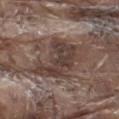The lesion was tiled from a total-body skin photograph and was not biopsied.
On the left thigh.
The tile uses white-light illumination.
About 6 mm across.
Cropped from a total-body skin-imaging series; the visible field is about 15 mm.
A male subject, roughly 80 years of age.